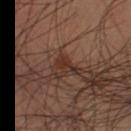Impression:
Imaged during a routine full-body skin examination; the lesion was not biopsied and no histopathology is available.
Image and clinical context:
Longest diameter approximately 3 mm. The total-body-photography lesion software estimated a classifier nevus-likeness of about 55/100 and a lesion-detection confidence of about 90/100. The tile uses cross-polarized illumination. From the left lower leg. A male subject, aged around 40. A roughly 15 mm field-of-view crop from a total-body skin photograph.